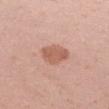This lesion was catalogued during total-body skin photography and was not selected for biopsy.
The lesion is located on the right forearm.
The subject is a female approximately 30 years of age.
Captured under white-light illumination.
The lesion-visualizer software estimated an area of roughly 8 mm², an outline eccentricity of about 0.55 (0 = round, 1 = elongated), and two-axis asymmetry of about 0.25. And it measured a lesion-detection confidence of about 100/100.
A region of skin cropped from a whole-body photographic capture, roughly 15 mm wide.
About 3.5 mm across.